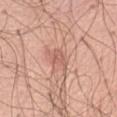{
  "biopsy_status": "not biopsied; imaged during a skin examination",
  "patient": {
    "sex": "male",
    "age_approx": 70
  },
  "site": "abdomen",
  "automated_metrics": {
    "border_irregularity_0_10": 3.0,
    "color_variation_0_10": 2.5,
    "peripheral_color_asymmetry": 1.0,
    "nevus_likeness_0_100": 0,
    "lesion_detection_confidence_0_100": 100
  },
  "image": {
    "source": "total-body photography crop",
    "field_of_view_mm": 15
  }
}